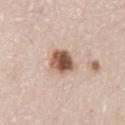Part of a total-body skin-imaging series; this lesion was reviewed on a skin check and was not flagged for biopsy. Automated tile analysis of the lesion measured an area of roughly 10 mm², a shape eccentricity near 0.5, and a symmetry-axis asymmetry near 0.2. The software also gave a mean CIELAB color near L≈58 a*≈17 b*≈28, about 16 CIELAB-L* units darker than the surrounding skin, and a lesion-to-skin contrast of about 10 (normalized; higher = more distinct). It also reported a within-lesion color-variation index near 8.5/10 and radial color variation of about 2.5. The analysis additionally found a nevus-likeness score of about 100/100 and lesion-presence confidence of about 100/100. A 15 mm crop from a total-body photograph taken for skin-cancer surveillance. Approximately 4 mm at its widest. From the left thigh. A male subject, about 80 years old. Captured under white-light illumination.